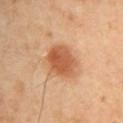<lesion>
<biopsy_status>not biopsied; imaged during a skin examination</biopsy_status>
<lighting>cross-polarized</lighting>
<site>left arm</site>
<image>
  <source>total-body photography crop</source>
  <field_of_view_mm>15</field_of_view_mm>
</image>
<patient>
  <sex>female</sex>
  <age_approx>40</age_approx>
</patient>
<lesion_size>
  <long_diameter_mm_approx>4.5</long_diameter_mm_approx>
</lesion_size>
<automated_metrics>
  <border_irregularity_0_10>2.0</border_irregularity_0_10>
  <nevus_likeness_0_100>100</nevus_likeness_0_100>
</automated_metrics>
</lesion>